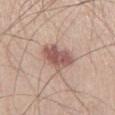Cropped from a total-body skin-imaging series; the visible field is about 15 mm. Approximately 4.5 mm at its widest. Located on the left thigh. A male subject aged 58–62. The total-body-photography lesion software estimated a shape eccentricity near 0.65 and two-axis asymmetry of about 0.25. The software also gave a nevus-likeness score of about 80/100 and a detector confidence of about 100 out of 100 that the crop contains a lesion.